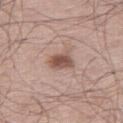This lesion was catalogued during total-body skin photography and was not selected for biopsy.
The lesion is on the leg.
This image is a 15 mm lesion crop taken from a total-body photograph.
Imaged with white-light lighting.
The recorded lesion diameter is about 3 mm.
A male subject, aged 58 to 62.